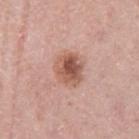Assessment: The lesion was photographed on a routine skin check and not biopsied; there is no pathology result. Clinical summary: Imaged with white-light lighting. From the leg. A female subject, roughly 40 years of age. Cropped from a whole-body photographic skin survey; the tile spans about 15 mm. The total-body-photography lesion software estimated a footprint of about 9.5 mm², an outline eccentricity of about 0.45 (0 = round, 1 = elongated), and two-axis asymmetry of about 0.15. And it measured an average lesion color of about L≈55 a*≈23 b*≈28 (CIELAB), roughly 13 lightness units darker than nearby skin, and a lesion-to-skin contrast of about 8.5 (normalized; higher = more distinct).